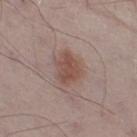{
  "biopsy_status": "not biopsied; imaged during a skin examination",
  "patient": {
    "sex": "male",
    "age_approx": 55
  },
  "image": {
    "source": "total-body photography crop",
    "field_of_view_mm": 15
  },
  "lighting": "white-light",
  "lesion_size": {
    "long_diameter_mm_approx": 4.0
  },
  "site": "leg",
  "automated_metrics": {
    "area_mm2_approx": 8.5,
    "eccentricity": 0.6,
    "cielab_L": 49,
    "cielab_a": 19,
    "cielab_b": 23,
    "vs_skin_darker_L": 9.0,
    "vs_skin_contrast_norm": 7.5,
    "border_irregularity_0_10": 3.0,
    "peripheral_color_asymmetry": 1.0
  }
}